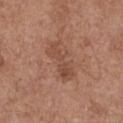{"biopsy_status": "not biopsied; imaged during a skin examination", "patient": {"sex": "female", "age_approx": 75}, "site": "front of the torso", "image": {"source": "total-body photography crop", "field_of_view_mm": 15}}